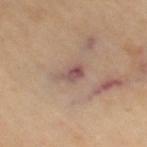notes = no biopsy performed (imaged during a skin exam)
subject = female, aged approximately 70
image = ~15 mm tile from a whole-body skin photo
body site = the right thigh